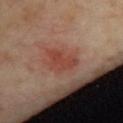No biopsy was performed on this lesion — it was imaged during a full skin examination and was not determined to be concerning.